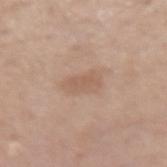Imaged during a routine full-body skin examination; the lesion was not biopsied and no histopathology is available. A 15 mm crop from a total-body photograph taken for skin-cancer surveillance. On the right upper arm. A male subject, in their mid- to late 50s. The tile uses white-light illumination. Measured at roughly 3.5 mm in maximum diameter.